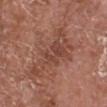Captured during whole-body skin photography for melanoma surveillance; the lesion was not biopsied. Imaged with white-light lighting. The lesion is located on the head or neck. A region of skin cropped from a whole-body photographic capture, roughly 15 mm wide. A male patient, aged 73–77. An algorithmic analysis of the crop reported an automated nevus-likeness rating near 0 out of 100 and a detector confidence of about 100 out of 100 that the crop contains a lesion.